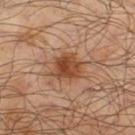On the right lower leg.
A lesion tile, about 15 mm wide, cut from a 3D total-body photograph.
The lesion's longest dimension is about 4 mm.
A male patient aged 63–67.
The lesion-visualizer software estimated a lesion area of about 9 mm², a shape eccentricity near 0.45, and two-axis asymmetry of about 0.25. The software also gave a peripheral color-asymmetry measure near 1.5.